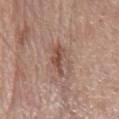<record>
  <biopsy_status>not biopsied; imaged during a skin examination</biopsy_status>
  <image>
    <source>total-body photography crop</source>
    <field_of_view_mm>15</field_of_view_mm>
  </image>
  <lighting>white-light</lighting>
  <site>chest</site>
  <lesion_size>
    <long_diameter_mm_approx>4.5</long_diameter_mm_approx>
  </lesion_size>
  <patient>
    <sex>male</sex>
    <age_approx>65</age_approx>
  </patient>
</record>